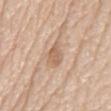No biopsy was performed on this lesion — it was imaged during a full skin examination and was not determined to be concerning. On the mid back. Automated tile analysis of the lesion measured an outline eccentricity of about 0.8 (0 = round, 1 = elongated). The software also gave an average lesion color of about L≈62 a*≈17 b*≈32 (CIELAB), roughly 9 lightness units darker than nearby skin, and a lesion-to-skin contrast of about 6 (normalized; higher = more distinct). And it measured internal color variation of about 2 on a 0–10 scale and a peripheral color-asymmetry measure near 0.5. The analysis additionally found a detector confidence of about 100 out of 100 that the crop contains a lesion. The recorded lesion diameter is about 3.5 mm. A male subject, in their mid-70s. A roughly 15 mm field-of-view crop from a total-body skin photograph. Imaged with white-light lighting.